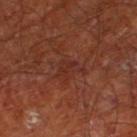Q: How was this image acquired?
A: total-body-photography crop, ~15 mm field of view
Q: What lighting was used for the tile?
A: cross-polarized
Q: What are the patient's age and sex?
A: male, aged 63 to 67
Q: Lesion location?
A: the left thigh